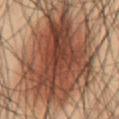Findings:
• workup · no biopsy performed (imaged during a skin exam)
• lighting · cross-polarized illumination
• imaging modality · 15 mm crop, total-body photography
• patient · male, approximately 55 years of age
• body site · the abdomen
• lesion diameter · ~11.5 mm (longest diameter)